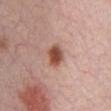The lesion was tiled from a total-body skin photograph and was not biopsied. Longest diameter approximately 2.5 mm. A male subject aged 28 to 32. This is a white-light tile. A close-up tile cropped from a whole-body skin photograph, about 15 mm across.